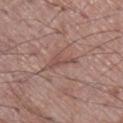Clinical impression: Part of a total-body skin-imaging series; this lesion was reviewed on a skin check and was not flagged for biopsy. Context: A roughly 15 mm field-of-view crop from a total-body skin photograph. The lesion is on the leg. The subject is a male approximately 70 years of age. About 3 mm across.